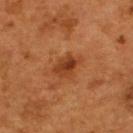{
  "biopsy_status": "not biopsied; imaged during a skin examination",
  "image": {
    "source": "total-body photography crop",
    "field_of_view_mm": 15
  },
  "automated_metrics": {
    "area_mm2_approx": 5.5,
    "eccentricity": 0.7,
    "shape_asymmetry": 0.3,
    "cielab_L": 37,
    "cielab_a": 26,
    "cielab_b": 35,
    "vs_skin_darker_L": 10.0,
    "vs_skin_contrast_norm": 8.0,
    "border_irregularity_0_10": 3.0,
    "color_variation_0_10": 5.5,
    "peripheral_color_asymmetry": 2.0,
    "nevus_likeness_0_100": 50,
    "lesion_detection_confidence_0_100": 100
  },
  "lesion_size": {
    "long_diameter_mm_approx": 3.0
  },
  "site": "back",
  "patient": {
    "sex": "male",
    "age_approx": 55
  }
}